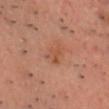This lesion was catalogued during total-body skin photography and was not selected for biopsy.
A male subject roughly 50 years of age.
Cropped from a whole-body photographic skin survey; the tile spans about 15 mm.
Captured under cross-polarized illumination.
Located on the chest.
An algorithmic analysis of the crop reported a normalized border contrast of about 5.5.
The recorded lesion diameter is about 3 mm.